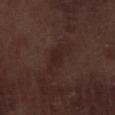workup = total-body-photography surveillance lesion; no biopsy
anatomic site = the right lower leg
patient = male, aged 68–72
image source = 15 mm crop, total-body photography